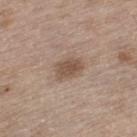Recorded during total-body skin imaging; not selected for excision or biopsy. A male patient aged approximately 70. A 15 mm close-up extracted from a 3D total-body photography capture. From the left thigh.